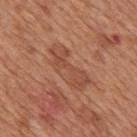Case summary:
- notes · total-body-photography surveillance lesion; no biopsy
- illumination · white-light
- acquisition · total-body-photography crop, ~15 mm field of view
- TBP lesion metrics · an area of roughly 12 mm², a shape eccentricity near 0.9, and a shape-asymmetry score of about 0.4 (0 = symmetric); a border-irregularity rating of about 5/10, internal color variation of about 3 on a 0–10 scale, and a peripheral color-asymmetry measure near 1; a detector confidence of about 100 out of 100 that the crop contains a lesion
- body site · the mid back
- patient · male, about 65 years old